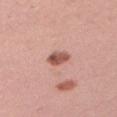No biopsy was performed on this lesion — it was imaged during a full skin examination and was not determined to be concerning.
Automated image analysis of the tile measured a footprint of about 4.5 mm², a shape eccentricity near 0.55, and a shape-asymmetry score of about 0.25 (0 = symmetric). The software also gave a lesion color around L≈55 a*≈25 b*≈26 in CIELAB, roughly 14 lightness units darker than nearby skin, and a lesion-to-skin contrast of about 9.5 (normalized; higher = more distinct). The software also gave border irregularity of about 2 on a 0–10 scale, a color-variation rating of about 4.5/10, and radial color variation of about 1.5. It also reported a lesion-detection confidence of about 100/100.
From the left upper arm.
Imaged with white-light lighting.
A male patient approximately 35 years of age.
A region of skin cropped from a whole-body photographic capture, roughly 15 mm wide.